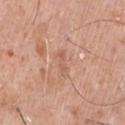Imaged during a routine full-body skin examination; the lesion was not biopsied and no histopathology is available. The lesion is located on the chest. Cropped from a whole-body photographic skin survey; the tile spans about 15 mm. A male patient, about 60 years old.